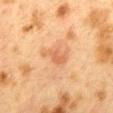The subject is a female aged around 40. Measured at roughly 3.5 mm in maximum diameter. The tile uses cross-polarized illumination. A 15 mm crop from a total-body photograph taken for skin-cancer surveillance. The lesion is located on the mid back. The total-body-photography lesion software estimated a symmetry-axis asymmetry near 0.35. The software also gave a lesion color around L≈53 a*≈21 b*≈34 in CIELAB, about 7 CIELAB-L* units darker than the surrounding skin, and a lesion-to-skin contrast of about 5.5 (normalized; higher = more distinct). The analysis additionally found a border-irregularity rating of about 4/10 and a within-lesion color-variation index near 2/10. The analysis additionally found a nevus-likeness score of about 0/100 and a detector confidence of about 100 out of 100 that the crop contains a lesion.